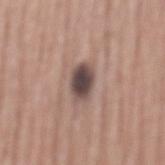<tbp_lesion>
<biopsy_status>not biopsied; imaged during a skin examination</biopsy_status>
<lighting>white-light</lighting>
<lesion_size>
  <long_diameter_mm_approx>4.0</long_diameter_mm_approx>
</lesion_size>
<site>mid back</site>
<image>
  <source>total-body photography crop</source>
  <field_of_view_mm>15</field_of_view_mm>
</image>
<patient>
  <sex>male</sex>
  <age_approx>75</age_approx>
</patient>
</tbp_lesion>